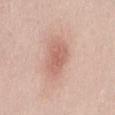{"biopsy_status": "not biopsied; imaged during a skin examination", "automated_metrics": {"cielab_L": 62, "cielab_a": 23, "cielab_b": 27, "vs_skin_darker_L": 10.0, "vs_skin_contrast_norm": 6.0}, "lesion_size": {"long_diameter_mm_approx": 4.0}, "image": {"source": "total-body photography crop", "field_of_view_mm": 15}, "site": "lower back", "patient": {"sex": "female", "age_approx": 20}}